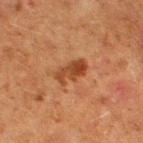- biopsy status · no biopsy performed (imaged during a skin exam)
- automated lesion analysis · an average lesion color of about L≈37 a*≈23 b*≈33 (CIELAB) and a lesion-to-skin contrast of about 8 (normalized; higher = more distinct); a border-irregularity index near 4/10, a color-variation rating of about 4.5/10, and peripheral color asymmetry of about 1.5; an automated nevus-likeness rating near 45 out of 100 and a detector confidence of about 100 out of 100 that the crop contains a lesion
- image · ~15 mm tile from a whole-body skin photo
- location · the right thigh
- patient · female, aged 48–52